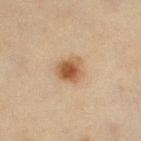<record>
  <biopsy_status>not biopsied; imaged during a skin examination</biopsy_status>
  <site>left lower leg</site>
  <lesion_size>
    <long_diameter_mm_approx>3.0</long_diameter_mm_approx>
  </lesion_size>
  <image>
    <source>total-body photography crop</source>
    <field_of_view_mm>15</field_of_view_mm>
  </image>
  <automated_metrics>
    <cielab_L>48</cielab_L>
    <cielab_a>18</cielab_a>
    <cielab_b>31</cielab_b>
    <vs_skin_darker_L>12.0</vs_skin_darker_L>
    <vs_skin_contrast_norm>9.0</vs_skin_contrast_norm>
    <border_irregularity_0_10>2.0</border_irregularity_0_10>
    <color_variation_0_10>5.0</color_variation_0_10>
    <peripheral_color_asymmetry>1.5</peripheral_color_asymmetry>
    <nevus_likeness_0_100>100</nevus_likeness_0_100>
  </automated_metrics>
  <lighting>cross-polarized</lighting>
  <patient>
    <sex>female</sex>
    <age_approx>45</age_approx>
  </patient>
</record>